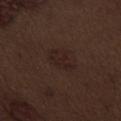• follow-up — total-body-photography surveillance lesion; no biopsy
• illumination — white-light illumination
• site — the abdomen
• image — total-body-photography crop, ~15 mm field of view
• lesion diameter — about 3 mm
• automated lesion analysis — a lesion color around L≈20 a*≈16 b*≈17 in CIELAB, about 4 CIELAB-L* units darker than the surrounding skin, and a normalized border contrast of about 6.5; a nevus-likeness score of about 5/100 and a lesion-detection confidence of about 100/100
• subject — male, aged around 70